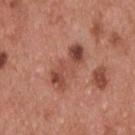Recorded during total-body skin imaging; not selected for excision or biopsy.
The lesion-visualizer software estimated roughly 10 lightness units darker than nearby skin and a normalized lesion–skin contrast near 7.5. The software also gave an automated nevus-likeness rating near 0 out of 100 and a lesion-detection confidence of about 100/100.
The recorded lesion diameter is about 5.5 mm.
The lesion is on the upper back.
A lesion tile, about 15 mm wide, cut from a 3D total-body photograph.
A male patient aged around 55.
Imaged with white-light lighting.